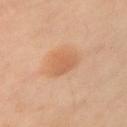Recorded during total-body skin imaging; not selected for excision or biopsy.
The lesion is on the left upper arm.
Cropped from a total-body skin-imaging series; the visible field is about 15 mm.
The subject is a female about 55 years old.
The tile uses cross-polarized illumination.
The total-body-photography lesion software estimated border irregularity of about 2.5 on a 0–10 scale, internal color variation of about 2 on a 0–10 scale, and a peripheral color-asymmetry measure near 0.5.
Measured at roughly 3 mm in maximum diameter.